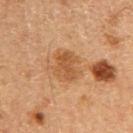Q: Is there a histopathology result?
A: imaged on a skin check; not biopsied
Q: Illumination type?
A: cross-polarized illumination
Q: Automated lesion metrics?
A: a mean CIELAB color near L≈47 a*≈20 b*≈35, a lesion–skin lightness drop of about 8, and a normalized border contrast of about 6.5
Q: What kind of image is this?
A: total-body-photography crop, ~15 mm field of view
Q: How large is the lesion?
A: ~4 mm (longest diameter)
Q: Who is the patient?
A: male, in their 60s